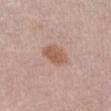Clinical impression:
This lesion was catalogued during total-body skin photography and was not selected for biopsy.
Acquisition and patient details:
A female subject, aged 63–67. Automated tile analysis of the lesion measured a lesion area of about 7.5 mm², an outline eccentricity of about 0.55 (0 = round, 1 = elongated), and two-axis asymmetry of about 0.2. The analysis additionally found a classifier nevus-likeness of about 80/100. Imaged with white-light lighting. The recorded lesion diameter is about 3.5 mm. A 15 mm close-up extracted from a 3D total-body photography capture. The lesion is on the abdomen.